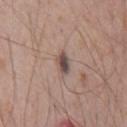notes = imaged on a skin check; not biopsied
diameter = about 2.5 mm
subject = male, aged approximately 70
lighting = white-light
image-analysis metrics = a footprint of about 4 mm², an eccentricity of roughly 0.75, and a symmetry-axis asymmetry near 0.2; an average lesion color of about L≈48 a*≈14 b*≈20 (CIELAB) and a normalized border contrast of about 9.5; a border-irregularity rating of about 1.5/10, a within-lesion color-variation index near 6.5/10, and peripheral color asymmetry of about 2.5; an automated nevus-likeness rating near 100 out of 100 and a detector confidence of about 100 out of 100 that the crop contains a lesion
site = the chest
image = total-body-photography crop, ~15 mm field of view